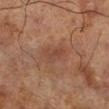Clinical impression:
Recorded during total-body skin imaging; not selected for excision or biopsy.
Background:
Automated image analysis of the tile measured a mean CIELAB color near L≈35 a*≈18 b*≈24 and a lesion–skin lightness drop of about 5. The software also gave radial color variation of about 1. It also reported a nevus-likeness score of about 0/100 and a lesion-detection confidence of about 100/100. A lesion tile, about 15 mm wide, cut from a 3D total-body photograph. Measured at roughly 3 mm in maximum diameter. The patient is a male in their 70s. This is a cross-polarized tile. The lesion is located on the right lower leg.